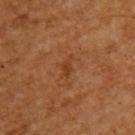Recorded during total-body skin imaging; not selected for excision or biopsy.
From the upper back.
A male subject, aged approximately 60.
A 15 mm close-up tile from a total-body photography series done for melanoma screening.